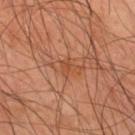Assessment: Captured during whole-body skin photography for melanoma surveillance; the lesion was not biopsied. Clinical summary: About 4 mm across. The lesion-visualizer software estimated a classifier nevus-likeness of about 0/100 and a detector confidence of about 90 out of 100 that the crop contains a lesion. Located on the mid back. A close-up tile cropped from a whole-body skin photograph, about 15 mm across. This is a cross-polarized tile. The subject is a male aged 43–47.